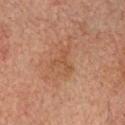Captured during whole-body skin photography for melanoma surveillance; the lesion was not biopsied. On the head or neck. A close-up tile cropped from a whole-body skin photograph, about 15 mm across. A male subject in their mid- to late 70s. Automated image analysis of the tile measured a lesion area of about 6 mm², a shape eccentricity near 0.65, and two-axis asymmetry of about 0.7. The software also gave a lesion color around L≈42 a*≈18 b*≈28 in CIELAB, roughly 5 lightness units darker than nearby skin, and a normalized lesion–skin contrast near 4.5. It also reported an automated nevus-likeness rating near 0 out of 100 and lesion-presence confidence of about 100/100. Imaged with cross-polarized lighting.